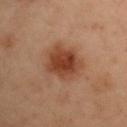| field | value |
|---|---|
| follow-up | total-body-photography surveillance lesion; no biopsy |
| subject | male, aged approximately 55 |
| site | the right upper arm |
| lighting | cross-polarized |
| imaging modality | total-body-photography crop, ~15 mm field of view |